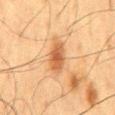| key | value |
|---|---|
| workup | imaged on a skin check; not biopsied |